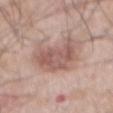The lesion was photographed on a routine skin check and not biopsied; there is no pathology result.
A male subject, approximately 60 years of age.
From the abdomen.
The total-body-photography lesion software estimated a footprint of about 17 mm², an outline eccentricity of about 0.65 (0 = round, 1 = elongated), and a shape-asymmetry score of about 0.35 (0 = symmetric). And it measured a mean CIELAB color near L≈56 a*≈20 b*≈24 and a normalized border contrast of about 7.
A close-up tile cropped from a whole-body skin photograph, about 15 mm across.
Captured under white-light illumination.
Longest diameter approximately 6.5 mm.